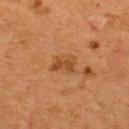The lesion was photographed on a routine skin check and not biopsied; there is no pathology result. The subject is a male in their mid- to late 50s. Cropped from a total-body skin-imaging series; the visible field is about 15 mm. Located on the back.